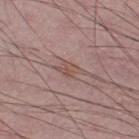notes — total-body-photography surveillance lesion; no biopsy
TBP lesion metrics — a footprint of about 4 mm², a shape eccentricity near 0.85, and a shape-asymmetry score of about 0.35 (0 = symmetric); border irregularity of about 4 on a 0–10 scale and peripheral color asymmetry of about 0.5
anatomic site — the right lower leg
patient — male, in their 50s
image source — 15 mm crop, total-body photography
lighting — white-light